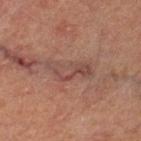• workup · catalogued during a skin exam; not biopsied
• tile lighting · cross-polarized illumination
• image source · ~15 mm crop, total-body skin-cancer survey
• TBP lesion metrics · a footprint of about 8.5 mm², an outline eccentricity of about 0.9 (0 = round, 1 = elongated), and a shape-asymmetry score of about 0.45 (0 = symmetric)
• diameter · ≈5 mm
• subject · male, aged approximately 60
• location · the left thigh